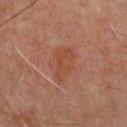Part of a total-body skin-imaging series; this lesion was reviewed on a skin check and was not flagged for biopsy. Approximately 4.5 mm at its widest. A male patient, aged around 60. Captured under cross-polarized illumination. From the chest. The lesion-visualizer software estimated an area of roughly 10 mm², an outline eccentricity of about 0.75 (0 = round, 1 = elongated), and a shape-asymmetry score of about 0.25 (0 = symmetric). A lesion tile, about 15 mm wide, cut from a 3D total-body photograph.